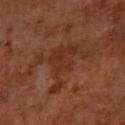notes = no biopsy performed (imaged during a skin exam); patient = female, in their mid- to late 60s; lesion size = ≈7 mm; lighting = cross-polarized; imaging modality = 15 mm crop, total-body photography; anatomic site = the left forearm.